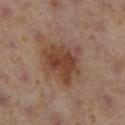This lesion was catalogued during total-body skin photography and was not selected for biopsy.
Located on the leg.
A lesion tile, about 15 mm wide, cut from a 3D total-body photograph.
A female subject aged 38–42.